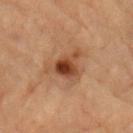lesion diameter = ≈4.5 mm
patient = male, aged around 85 or older
acquisition = ~15 mm tile from a whole-body skin photo
lighting = cross-polarized
location = the left forearm